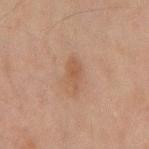Q: Is there a histopathology result?
A: catalogued during a skin exam; not biopsied
Q: How was this image acquired?
A: total-body-photography crop, ~15 mm field of view
Q: Automated lesion metrics?
A: an area of roughly 4.5 mm², an eccentricity of roughly 0.9, and a shape-asymmetry score of about 0.3 (0 = symmetric); an average lesion color of about L≈43 a*≈17 b*≈26 (CIELAB), roughly 6 lightness units darker than nearby skin, and a normalized lesion–skin contrast near 6; a nevus-likeness score of about 0/100 and a detector confidence of about 100 out of 100 that the crop contains a lesion
Q: What is the lesion's diameter?
A: ≈4 mm
Q: What is the anatomic site?
A: the mid back
Q: What lighting was used for the tile?
A: cross-polarized
Q: Who is the patient?
A: male, aged 43 to 47